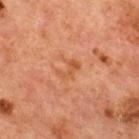Part of a total-body skin-imaging series; this lesion was reviewed on a skin check and was not flagged for biopsy.
Automated tile analysis of the lesion measured a lesion area of about 3.5 mm² and an outline eccentricity of about 0.8 (0 = round, 1 = elongated). It also reported an average lesion color of about L≈43 a*≈22 b*≈33 (CIELAB) and a lesion-to-skin contrast of about 6 (normalized; higher = more distinct).
Measured at roughly 2.5 mm in maximum diameter.
Captured under cross-polarized illumination.
On the chest.
The subject is a male aged approximately 65.
A close-up tile cropped from a whole-body skin photograph, about 15 mm across.